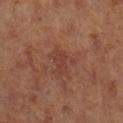TBP lesion metrics: a lesion color around L≈41 a*≈25 b*≈28 in CIELAB, about 6 CIELAB-L* units darker than the surrounding skin, and a normalized lesion–skin contrast near 5; a border-irregularity rating of about 3.5/10, internal color variation of about 1.5 on a 0–10 scale, and a peripheral color-asymmetry measure near 0.5; an automated nevus-likeness rating near 5 out of 100 and a detector confidence of about 100 out of 100 that the crop contains a lesion | location: the leg | tile lighting: cross-polarized illumination | size: about 2.5 mm | image source: total-body-photography crop, ~15 mm field of view | patient: female.